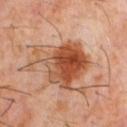notes = catalogued during a skin exam; not biopsied
subject = male, roughly 60 years of age
lighting = cross-polarized illumination
image-analysis metrics = a lesion–skin lightness drop of about 12 and a normalized border contrast of about 9.5; a nevus-likeness score of about 90/100 and a lesion-detection confidence of about 100/100
acquisition = total-body-photography crop, ~15 mm field of view
location = the chest